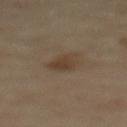Imaged during a routine full-body skin examination; the lesion was not biopsied and no histopathology is available. Automated tile analysis of the lesion measured a footprint of about 6 mm², a shape eccentricity near 0.6, and two-axis asymmetry of about 0.25. It also reported a mean CIELAB color near L≈38 a*≈13 b*≈26, about 7 CIELAB-L* units darker than the surrounding skin, and a normalized lesion–skin contrast near 6.5. And it measured a border-irregularity rating of about 2.5/10 and peripheral color asymmetry of about 1. The analysis additionally found a classifier nevus-likeness of about 35/100 and a lesion-detection confidence of about 100/100. A region of skin cropped from a whole-body photographic capture, roughly 15 mm wide. A female subject about 60 years old. From the upper back. The tile uses cross-polarized illumination.